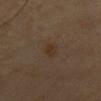Impression:
This lesion was catalogued during total-body skin photography and was not selected for biopsy.